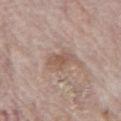Q: Was a biopsy performed?
A: no biopsy performed (imaged during a skin exam)
Q: What is the anatomic site?
A: the mid back
Q: What are the patient's age and sex?
A: male, aged 68–72
Q: How was this image acquired?
A: ~15 mm tile from a whole-body skin photo
Q: Automated lesion metrics?
A: an average lesion color of about L≈54 a*≈17 b*≈26 (CIELAB) and a lesion-to-skin contrast of about 6.5 (normalized; higher = more distinct); a lesion-detection confidence of about 100/100
Q: How was the tile lit?
A: white-light